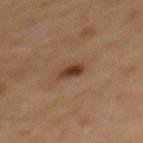Q: Was a biopsy performed?
A: catalogued during a skin exam; not biopsied
Q: Illumination type?
A: cross-polarized illumination
Q: Automated lesion metrics?
A: a lesion area of about 4 mm², an eccentricity of roughly 0.75, and two-axis asymmetry of about 0.25; a detector confidence of about 100 out of 100 that the crop contains a lesion
Q: Lesion location?
A: the mid back
Q: Patient demographics?
A: male, roughly 65 years of age
Q: Lesion size?
A: ~2.5 mm (longest diameter)
Q: What kind of image is this?
A: ~15 mm tile from a whole-body skin photo